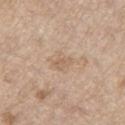This lesion was catalogued during total-body skin photography and was not selected for biopsy.
Cropped from a total-body skin-imaging series; the visible field is about 15 mm.
The patient is a male aged 68 to 72.
From the left thigh.
The lesion's longest dimension is about 3 mm.
Automated image analysis of the tile measured a lesion area of about 4 mm² and an eccentricity of roughly 0.75. The software also gave a lesion color around L≈61 a*≈15 b*≈30 in CIELAB, a lesion–skin lightness drop of about 6, and a normalized border contrast of about 4.5. And it measured border irregularity of about 3.5 on a 0–10 scale, a color-variation rating of about 2/10, and radial color variation of about 0.5. And it measured a lesion-detection confidence of about 100/100.